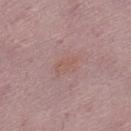<tbp_lesion>
<biopsy_status>not biopsied; imaged during a skin examination</biopsy_status>
<lighting>white-light</lighting>
<lesion_size>
  <long_diameter_mm_approx>3.0</long_diameter_mm_approx>
</lesion_size>
<site>left lower leg</site>
<image>
  <source>total-body photography crop</source>
  <field_of_view_mm>15</field_of_view_mm>
</image>
<patient>
  <sex>female</sex>
  <age_approx>50</age_approx>
</patient>
</tbp_lesion>